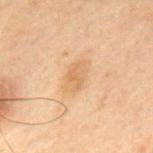workup: catalogued during a skin exam; not biopsied | illumination: cross-polarized illumination | body site: the upper back | lesion diameter: ~3.5 mm (longest diameter) | patient: male, aged approximately 70 | acquisition: 15 mm crop, total-body photography.